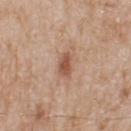| key | value |
|---|---|
| workup | no biopsy performed (imaged during a skin exam) |
| site | the upper back |
| lighting | white-light |
| imaging modality | total-body-photography crop, ~15 mm field of view |
| lesion diameter | about 3 mm |
| subject | male, aged 78–82 |